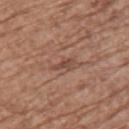The lesion was tiled from a total-body skin photograph and was not biopsied. A lesion tile, about 15 mm wide, cut from a 3D total-body photograph. Measured at roughly 2.5 mm in maximum diameter. The total-body-photography lesion software estimated a classifier nevus-likeness of about 0/100 and a detector confidence of about 90 out of 100 that the crop contains a lesion. Captured under white-light illumination. A female patient about 75 years old. From the back.